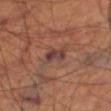illumination: cross-polarized
automated lesion analysis: a footprint of about 4.5 mm² and a shape-asymmetry score of about 0.3 (0 = symmetric); a lesion color around L≈38 a*≈19 b*≈21 in CIELAB, roughly 9 lightness units darker than nearby skin, and a normalized lesion–skin contrast near 9; a border-irregularity rating of about 3/10; a classifier nevus-likeness of about 0/100
size: ≈3 mm
image: ~15 mm crop, total-body skin-cancer survey
subject: male, approximately 70 years of age
body site: the leg